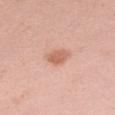Assessment:
The lesion was photographed on a routine skin check and not biopsied; there is no pathology result.
Background:
A female patient, aged around 30. The lesion is on the left forearm. This image is a 15 mm lesion crop taken from a total-body photograph. Measured at roughly 3 mm in maximum diameter. The tile uses white-light illumination. The lesion-visualizer software estimated a lesion–skin lightness drop of about 10 and a normalized border contrast of about 7. It also reported border irregularity of about 2 on a 0–10 scale and a within-lesion color-variation index near 2/10.